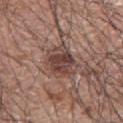Assessment:
Recorded during total-body skin imaging; not selected for excision or biopsy.
Image and clinical context:
A region of skin cropped from a whole-body photographic capture, roughly 15 mm wide. Captured under white-light illumination. A male patient, aged approximately 70. Longest diameter approximately 4 mm. Located on the left arm.